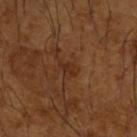Findings:
– follow-up — total-body-photography surveillance lesion; no biopsy
– tile lighting — cross-polarized
– anatomic site — the right forearm
– lesion size — about 2.5 mm
– imaging modality — ~15 mm crop, total-body skin-cancer survey
– subject — male, approximately 65 years of age